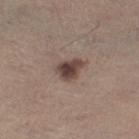Clinical impression:
Recorded during total-body skin imaging; not selected for excision or biopsy.
Clinical summary:
The tile uses white-light illumination. On the right thigh. The patient is a female aged around 65. Automated image analysis of the tile measured a lesion area of about 6.5 mm², an eccentricity of roughly 0.65, and a symmetry-axis asymmetry near 0.35. And it measured an average lesion color of about L≈43 a*≈16 b*≈21 (CIELAB), a lesion–skin lightness drop of about 14, and a lesion-to-skin contrast of about 10.5 (normalized; higher = more distinct). Measured at roughly 3.5 mm in maximum diameter. A close-up tile cropped from a whole-body skin photograph, about 15 mm across.